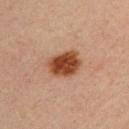notes = total-body-photography surveillance lesion; no biopsy
patient = male, aged around 30
image source = total-body-photography crop, ~15 mm field of view
site = the left upper arm
lesion size = about 4.5 mm